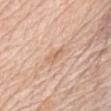Background:
The patient is a male aged around 80. On the chest. Captured under white-light illumination. A 15 mm crop from a total-body photograph taken for skin-cancer surveillance.